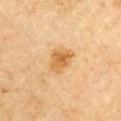Recorded during total-body skin imaging; not selected for excision or biopsy. On the left upper arm. The subject is a male approximately 65 years of age. A 15 mm crop from a total-body photograph taken for skin-cancer surveillance.